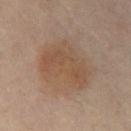| feature | finding |
|---|---|
| follow-up | catalogued during a skin exam; not biopsied |
| diameter | ~7 mm (longest diameter) |
| image source | ~15 mm crop, total-body skin-cancer survey |
| illumination | cross-polarized illumination |
| site | the abdomen |
| subject | male, in their 60s |